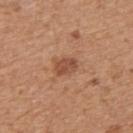Q: Was a biopsy performed?
A: catalogued during a skin exam; not biopsied
Q: What are the patient's age and sex?
A: male, aged around 55
Q: What is the lesion's diameter?
A: ~2.5 mm (longest diameter)
Q: What kind of image is this?
A: ~15 mm crop, total-body skin-cancer survey
Q: Illumination type?
A: white-light
Q: What is the anatomic site?
A: the right upper arm